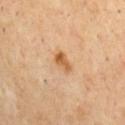Findings:
– body site: the chest
– illumination: cross-polarized
– imaging modality: ~15 mm crop, total-body skin-cancer survey
– lesion size: about 3 mm
– patient: male, aged 68–72